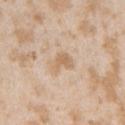| field | value |
|---|---|
| biopsy status | catalogued during a skin exam; not biopsied |
| size | ~2.5 mm (longest diameter) |
| tile lighting | white-light |
| location | the left upper arm |
| image source | 15 mm crop, total-body photography |
| subject | female, aged approximately 25 |